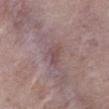Clinical impression:
Part of a total-body skin-imaging series; this lesion was reviewed on a skin check and was not flagged for biopsy.
Clinical summary:
The lesion is located on the abdomen. Captured under white-light illumination. A male patient aged 58–62. The lesion's longest dimension is about 3 mm. A 15 mm close-up tile from a total-body photography series done for melanoma screening.